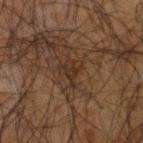Clinical impression:
Imaged during a routine full-body skin examination; the lesion was not biopsied and no histopathology is available.
Clinical summary:
The lesion is located on the right upper arm. About 3 mm across. A male subject, aged around 65. Automated tile analysis of the lesion measured an area of roughly 4 mm² and a symmetry-axis asymmetry near 0.65. And it measured an average lesion color of about L≈24 a*≈14 b*≈22 (CIELAB) and a lesion–skin lightness drop of about 5. A close-up tile cropped from a whole-body skin photograph, about 15 mm across. Captured under cross-polarized illumination.